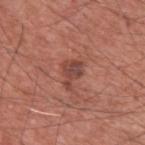Captured during whole-body skin photography for melanoma surveillance; the lesion was not biopsied. Measured at roughly 3 mm in maximum diameter. A close-up tile cropped from a whole-body skin photograph, about 15 mm across. A male patient about 60 years old. Captured under white-light illumination. Located on the upper back.